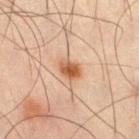Captured during whole-body skin photography for melanoma surveillance; the lesion was not biopsied. This image is a 15 mm lesion crop taken from a total-body photograph. A male subject, aged approximately 45. The total-body-photography lesion software estimated a border-irregularity index near 2/10. The software also gave a nevus-likeness score of about 95/100 and a lesion-detection confidence of about 100/100. Located on the right thigh. Captured under cross-polarized illumination.